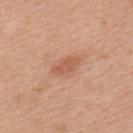| feature | finding |
|---|---|
| location | the upper back |
| imaging modality | ~15 mm tile from a whole-body skin photo |
| lighting | white-light |
| subject | female, about 40 years old |
| diameter | about 3.5 mm |